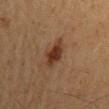* notes — imaged on a skin check; not biopsied
* TBP lesion metrics — a border-irregularity rating of about 3/10, a within-lesion color-variation index near 3/10, and radial color variation of about 1; an automated nevus-likeness rating near 95 out of 100 and a lesion-detection confidence of about 100/100
* tile lighting — cross-polarized illumination
* patient — male, approximately 60 years of age
* lesion size — ~4 mm (longest diameter)
* body site — the mid back
* imaging modality — 15 mm crop, total-body photography